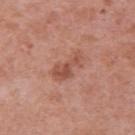Cropped from a whole-body photographic skin survey; the tile spans about 15 mm. The subject is a female aged around 40. The tile uses white-light illumination. An algorithmic analysis of the crop reported a color-variation rating of about 2.5/10 and radial color variation of about 1. And it measured a nevus-likeness score of about 5/100. The lesion is on the left upper arm.